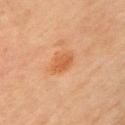workup: catalogued during a skin exam; not biopsied
patient: female, aged approximately 70
size: ~3.5 mm (longest diameter)
image: 15 mm crop, total-body photography
location: the left upper arm
lighting: cross-polarized